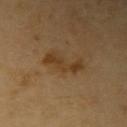This lesion was catalogued during total-body skin photography and was not selected for biopsy. The lesion is located on the arm. A male patient, aged 63 to 67. The lesion's longest dimension is about 5 mm. A close-up tile cropped from a whole-body skin photograph, about 15 mm across.